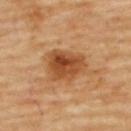This lesion was catalogued during total-body skin photography and was not selected for biopsy. Located on the upper back. A lesion tile, about 15 mm wide, cut from a 3D total-body photograph. The subject is a female aged approximately 60. The total-body-photography lesion software estimated a footprint of about 14 mm², a shape eccentricity near 0.6, and two-axis asymmetry of about 0.25. The software also gave a border-irregularity rating of about 2.5/10, a color-variation rating of about 7/10, and peripheral color asymmetry of about 2.5.